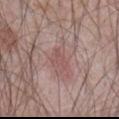Recorded during total-body skin imaging; not selected for excision or biopsy. A 15 mm close-up extracted from a 3D total-body photography capture. On the abdomen. A male subject aged approximately 65.